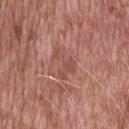Recorded during total-body skin imaging; not selected for excision or biopsy.
The tile uses white-light illumination.
Approximately 4 mm at its widest.
A male patient, about 50 years old.
Located on the head or neck.
Cropped from a whole-body photographic skin survey; the tile spans about 15 mm.